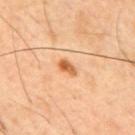Part of a total-body skin-imaging series; this lesion was reviewed on a skin check and was not flagged for biopsy.
The tile uses cross-polarized illumination.
The lesion is on the mid back.
A male subject, aged approximately 45.
The recorded lesion diameter is about 2.5 mm.
This image is a 15 mm lesion crop taken from a total-body photograph.